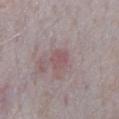Q: How was this image acquired?
A: 15 mm crop, total-body photography
Q: What is the lesion's diameter?
A: ~3 mm (longest diameter)
Q: Who is the patient?
A: male, aged approximately 65
Q: What is the anatomic site?
A: the chest
Q: How was the tile lit?
A: white-light illumination